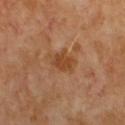Impression:
Recorded during total-body skin imaging; not selected for excision or biopsy.
Background:
A region of skin cropped from a whole-body photographic capture, roughly 15 mm wide. A male subject, approximately 65 years of age. The tile uses cross-polarized illumination. Automated tile analysis of the lesion measured border irregularity of about 3 on a 0–10 scale, internal color variation of about 1 on a 0–10 scale, and radial color variation of about 0.5. Measured at roughly 2.5 mm in maximum diameter.